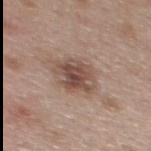Case summary:
* biopsy status: no biopsy performed (imaged during a skin exam)
* illumination: white-light
* anatomic site: the upper back
* patient: female, aged around 40
* imaging modality: ~15 mm tile from a whole-body skin photo
* size: ~4 mm (longest diameter)